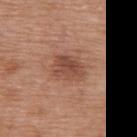Assessment:
Recorded during total-body skin imaging; not selected for excision or biopsy.
Context:
The lesion is located on the upper back. About 3.5 mm across. A female subject aged approximately 70. The tile uses white-light illumination. A lesion tile, about 15 mm wide, cut from a 3D total-body photograph.